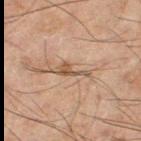This lesion was catalogued during total-body skin photography and was not selected for biopsy. The tile uses cross-polarized illumination. Automated tile analysis of the lesion measured an area of roughly 3.5 mm², a shape eccentricity near 0.95, and a shape-asymmetry score of about 0.55 (0 = symmetric). And it measured an average lesion color of about L≈43 a*≈15 b*≈26 (CIELAB), roughly 9 lightness units darker than nearby skin, and a normalized lesion–skin contrast near 7.5. A 15 mm crop from a total-body photograph taken for skin-cancer surveillance. Approximately 4 mm at its widest. Located on the right thigh. A male subject roughly 65 years of age.